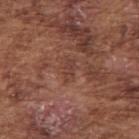<case>
<biopsy_status>not biopsied; imaged during a skin examination</biopsy_status>
<site>right upper arm</site>
<patient>
  <sex>male</sex>
  <age_approx>75</age_approx>
</patient>
<automated_metrics>
  <cielab_L>40</cielab_L>
  <cielab_a>22</cielab_a>
  <cielab_b>26</cielab_b>
  <vs_skin_darker_L>6.0</vs_skin_darker_L>
  <vs_skin_contrast_norm>5.5</vs_skin_contrast_norm>
  <border_irregularity_0_10>3.0</border_irregularity_0_10>
  <color_variation_0_10>0.0</color_variation_0_10>
  <peripheral_color_asymmetry>0.0</peripheral_color_asymmetry>
  <nevus_likeness_0_100>0</nevus_likeness_0_100>
  <lesion_detection_confidence_0_100>100</lesion_detection_confidence_0_100>
</automated_metrics>
<lighting>white-light</lighting>
<image>
  <source>total-body photography crop</source>
  <field_of_view_mm>15</field_of_view_mm>
</image>
</case>